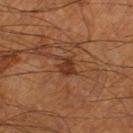Imaged during a routine full-body skin examination; the lesion was not biopsied and no histopathology is available. This image is a 15 mm lesion crop taken from a total-body photograph. About 3 mm across. From the left leg. A male patient, in their mid- to late 60s. Captured under cross-polarized illumination.